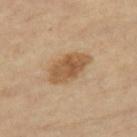Recorded during total-body skin imaging; not selected for excision or biopsy.
The lesion is located on the right thigh.
A 15 mm close-up extracted from a 3D total-body photography capture.
An algorithmic analysis of the crop reported a footprint of about 12 mm² and a shape eccentricity near 0.85. The software also gave a lesion color around L≈56 a*≈18 b*≈36 in CIELAB, roughly 11 lightness units darker than nearby skin, and a normalized lesion–skin contrast near 8. The software also gave internal color variation of about 4 on a 0–10 scale.
A female subject, aged 63–67.